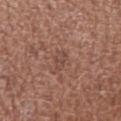The lesion was tiled from a total-body skin photograph and was not biopsied. The lesion is on the right upper arm. A female subject aged 48–52. Cropped from a total-body skin-imaging series; the visible field is about 15 mm. The recorded lesion diameter is about 3 mm.